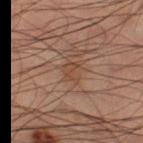This lesion was catalogued during total-body skin photography and was not selected for biopsy.
A male subject, aged around 60.
From the left thigh.
Cropped from a whole-body photographic skin survey; the tile spans about 15 mm.